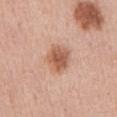biopsy status — imaged on a skin check; not biopsied | lesion diameter — ≈3.5 mm | location — the abdomen | image — ~15 mm crop, total-body skin-cancer survey | TBP lesion metrics — a mean CIELAB color near L≈58 a*≈23 b*≈32, roughly 12 lightness units darker than nearby skin, and a lesion-to-skin contrast of about 8.5 (normalized; higher = more distinct); border irregularity of about 1.5 on a 0–10 scale, internal color variation of about 4 on a 0–10 scale, and a peripheral color-asymmetry measure near 1 | patient — male, aged approximately 70 | lighting — white-light illumination.